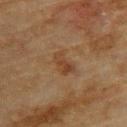Impression: Imaged during a routine full-body skin examination; the lesion was not biopsied and no histopathology is available.